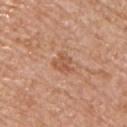workup: no biopsy performed (imaged during a skin exam) | anatomic site: the chest | lesion size: ~3 mm (longest diameter) | subject: male, roughly 70 years of age | acquisition: 15 mm crop, total-body photography.